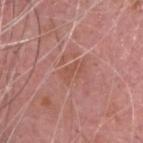Clinical impression: Recorded during total-body skin imaging; not selected for excision or biopsy. Acquisition and patient details: A region of skin cropped from a whole-body photographic capture, roughly 15 mm wide. The total-body-photography lesion software estimated an average lesion color of about L≈52 a*≈25 b*≈28 (CIELAB), a lesion–skin lightness drop of about 6, and a normalized lesion–skin contrast near 5.5. The analysis additionally found border irregularity of about 5 on a 0–10 scale, a color-variation rating of about 0.5/10, and radial color variation of about 0. This is a white-light tile. The patient is a male in their mid-70s. Approximately 3 mm at its widest. Located on the head or neck.